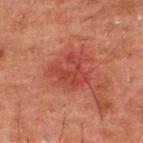{"biopsy_status": "not biopsied; imaged during a skin examination", "patient": {"sex": "male", "age_approx": 50}, "site": "upper back", "image": {"source": "total-body photography crop", "field_of_view_mm": 15}}